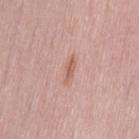Findings:
- image · 15 mm crop, total-body photography
- site · the back
- patient · female, roughly 50 years of age
- size · about 2.5 mm